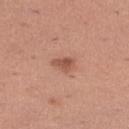Q: Was a biopsy performed?
A: catalogued during a skin exam; not biopsied
Q: What is the anatomic site?
A: the leg
Q: What lighting was used for the tile?
A: white-light
Q: How was this image acquired?
A: 15 mm crop, total-body photography
Q: Who is the patient?
A: female, approximately 30 years of age